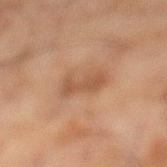This lesion was catalogued during total-body skin photography and was not selected for biopsy. A 15 mm crop from a total-body photograph taken for skin-cancer surveillance. Imaged with cross-polarized lighting. The total-body-photography lesion software estimated a mean CIELAB color near L≈42 a*≈18 b*≈27 and a lesion-to-skin contrast of about 6 (normalized; higher = more distinct). The software also gave internal color variation of about 1 on a 0–10 scale. The software also gave a lesion-detection confidence of about 100/100. The subject is a male aged approximately 45. From the left lower leg.